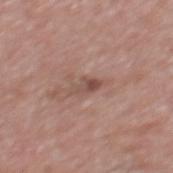notes — no biopsy performed (imaged during a skin exam)
patient — male, in their 70s
lesion size — about 3.5 mm
image source — 15 mm crop, total-body photography
location — the mid back
lighting — white-light illumination
automated lesion analysis — a symmetry-axis asymmetry near 0.35; a lesion color around L≈49 a*≈20 b*≈24 in CIELAB and roughly 9 lightness units darker than nearby skin; a border-irregularity rating of about 4.5/10 and a color-variation rating of about 2.5/10